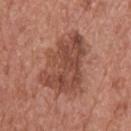follow-up = no biopsy performed (imaged during a skin exam) | lesion diameter = ≈7.5 mm | tile lighting = white-light | subject = male, roughly 70 years of age | acquisition = ~15 mm crop, total-body skin-cancer survey | TBP lesion metrics = a nevus-likeness score of about 15/100 and lesion-presence confidence of about 100/100 | location = the upper back.